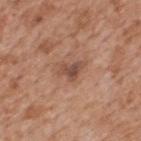The tile uses white-light illumination. A region of skin cropped from a whole-body photographic capture, roughly 15 mm wide. A male subject, aged 63 to 67. On the upper back. Approximately 3 mm at its widest.